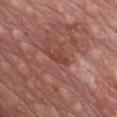notes — no biopsy performed (imaged during a skin exam)
body site — the chest
image — ~15 mm tile from a whole-body skin photo
patient — male, approximately 60 years of age